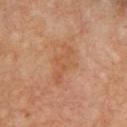Clinical impression:
Imaged during a routine full-body skin examination; the lesion was not biopsied and no histopathology is available.
Image and clinical context:
The lesion is on the upper back. The subject is a female aged 63–67. A roughly 15 mm field-of-view crop from a total-body skin photograph.